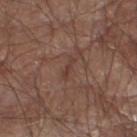follow-up: total-body-photography surveillance lesion; no biopsy
tile lighting: white-light
imaging modality: ~15 mm crop, total-body skin-cancer survey
subject: male, approximately 80 years of age
anatomic site: the left lower leg